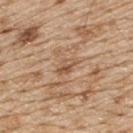Assessment: No biopsy was performed on this lesion — it was imaged during a full skin examination and was not determined to be concerning. Context: The total-body-photography lesion software estimated an outline eccentricity of about 0.8 (0 = round, 1 = elongated) and a shape-asymmetry score of about 0.25 (0 = symmetric). It also reported a lesion–skin lightness drop of about 9 and a normalized lesion–skin contrast near 6.5. And it measured a border-irregularity rating of about 3/10 and radial color variation of about 1.5. The analysis additionally found a nevus-likeness score of about 0/100 and a detector confidence of about 65 out of 100 that the crop contains a lesion. The lesion is located on the back. A roughly 15 mm field-of-view crop from a total-body skin photograph. This is a white-light tile. About 2.5 mm across. The patient is a male aged approximately 70.